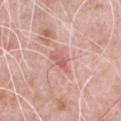Assessment: This lesion was catalogued during total-body skin photography and was not selected for biopsy. Background: The lesion is located on the chest. Cropped from a total-body skin-imaging series; the visible field is about 15 mm. A male patient, aged approximately 50.